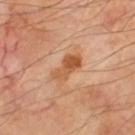| key | value |
|---|---|
| workup | imaged on a skin check; not biopsied |
| tile lighting | cross-polarized illumination |
| image source | total-body-photography crop, ~15 mm field of view |
| subject | male, aged 68–72 |
| lesion diameter | about 4 mm |
| body site | the left thigh |